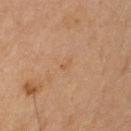This lesion was catalogued during total-body skin photography and was not selected for biopsy.
A region of skin cropped from a whole-body photographic capture, roughly 15 mm wide.
Automated tile analysis of the lesion measured an eccentricity of roughly 0.85. The analysis additionally found a mean CIELAB color near L≈54 a*≈20 b*≈33. It also reported border irregularity of about 4 on a 0–10 scale, a color-variation rating of about 0/10, and peripheral color asymmetry of about 0.
Longest diameter approximately 1.5 mm.
From the left upper arm.
A female subject, aged 63 to 67.
Captured under cross-polarized illumination.